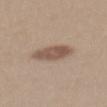<lesion>
<biopsy_status>not biopsied; imaged during a skin examination</biopsy_status>
<lesion_size>
  <long_diameter_mm_approx>3.5</long_diameter_mm_approx>
</lesion_size>
<image>
  <source>total-body photography crop</source>
  <field_of_view_mm>15</field_of_view_mm>
</image>
<patient>
  <sex>female</sex>
  <age_approx>30</age_approx>
</patient>
<site>upper back</site>
<lighting>white-light</lighting>
</lesion>